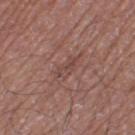Part of a total-body skin-imaging series; this lesion was reviewed on a skin check and was not flagged for biopsy.
A close-up tile cropped from a whole-body skin photograph, about 15 mm across.
The lesion is located on the left thigh.
A male patient roughly 70 years of age.